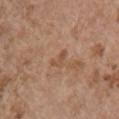{
  "biopsy_status": "not biopsied; imaged during a skin examination",
  "lighting": "white-light",
  "image": {
    "source": "total-body photography crop",
    "field_of_view_mm": 15
  },
  "patient": {
    "sex": "female",
    "age_approx": 65
  },
  "site": "right upper arm"
}